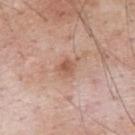workup: total-body-photography surveillance lesion; no biopsy
location: the upper back
image source: total-body-photography crop, ~15 mm field of view
patient: male, aged around 60
lighting: white-light